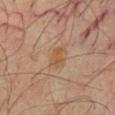  biopsy_status: not biopsied; imaged during a skin examination